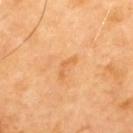The lesion was tiled from a total-body skin photograph and was not biopsied. The lesion-visualizer software estimated a lesion area of about 2 mm². The software also gave a border-irregularity rating of about 8/10 and radial color variation of about 0. Cropped from a total-body skin-imaging series; the visible field is about 15 mm. The lesion is located on the upper back. The patient is a male aged approximately 70. Imaged with cross-polarized lighting. The recorded lesion diameter is about 3 mm.